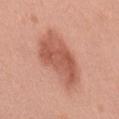biopsy status — no biopsy performed (imaged during a skin exam) | location — the mid back | diameter — ~7.5 mm (longest diameter) | imaging modality — ~15 mm crop, total-body skin-cancer survey | patient — female, aged around 40.